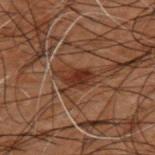– workup · total-body-photography surveillance lesion; no biopsy
– imaging modality · 15 mm crop, total-body photography
– patient · male, aged around 50
– anatomic site · the upper back
– tile lighting · cross-polarized
– TBP lesion metrics · a lesion area of about 6 mm², an eccentricity of roughly 0.8, and a symmetry-axis asymmetry near 0.25; about 8 CIELAB-L* units darker than the surrounding skin and a normalized border contrast of about 8.5; border irregularity of about 3 on a 0–10 scale